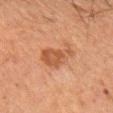workup — imaged on a skin check; not biopsied
anatomic site — the left forearm
acquisition — 15 mm crop, total-body photography
patient — female, about 60 years old